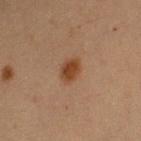Assessment:
No biopsy was performed on this lesion — it was imaged during a full skin examination and was not determined to be concerning.
Acquisition and patient details:
The lesion is on the arm. Cropped from a whole-body photographic skin survey; the tile spans about 15 mm. The subject is a female aged around 40.